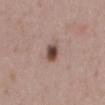Q: Was a biopsy performed?
A: catalogued during a skin exam; not biopsied
Q: What is the anatomic site?
A: the mid back
Q: How large is the lesion?
A: ~2.5 mm (longest diameter)
Q: How was the tile lit?
A: white-light illumination
Q: Automated lesion metrics?
A: an outline eccentricity of about 0.75 (0 = round, 1 = elongated) and two-axis asymmetry of about 0.2; border irregularity of about 1.5 on a 0–10 scale and radial color variation of about 2
Q: How was this image acquired?
A: 15 mm crop, total-body photography
Q: What are the patient's age and sex?
A: female, in their 30s